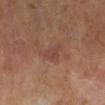{"biopsy_status": "not biopsied; imaged during a skin examination"}